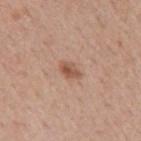follow-up — total-body-photography surveillance lesion; no biopsy | image source — 15 mm crop, total-body photography | lighting — white-light illumination | diameter — about 2.5 mm | subject — male, in their mid- to late 50s | body site — the back.